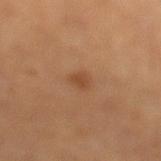Q: Was this lesion biopsied?
A: catalogued during a skin exam; not biopsied
Q: How was the tile lit?
A: cross-polarized
Q: How was this image acquired?
A: total-body-photography crop, ~15 mm field of view
Q: Where on the body is the lesion?
A: the left lower leg
Q: Lesion size?
A: ~2 mm (longest diameter)
Q: What are the patient's age and sex?
A: female, approximately 65 years of age